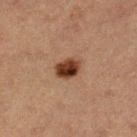The lesion was tiled from a total-body skin photograph and was not biopsied.
The tile uses cross-polarized illumination.
Approximately 3.5 mm at its widest.
The lesion is located on the left lower leg.
The subject is a female in their 40s.
Cropped from a whole-body photographic skin survey; the tile spans about 15 mm.